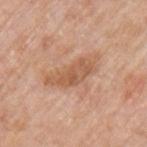biopsy status: imaged on a skin check; not biopsied.